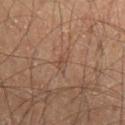Findings:
• follow-up · no biopsy performed (imaged during a skin exam)
• subject · male, about 55 years old
• body site · the mid back
• image source · ~15 mm tile from a whole-body skin photo
• lesion diameter · ≈3 mm